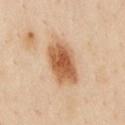Part of a total-body skin-imaging series; this lesion was reviewed on a skin check and was not flagged for biopsy.
A roughly 15 mm field-of-view crop from a total-body skin photograph.
The patient is a male aged around 55.
The lesion is on the front of the torso.